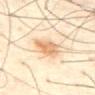follow-up — total-body-photography surveillance lesion; no biopsy | acquisition — ~15 mm crop, total-body skin-cancer survey | subject — male, aged approximately 40 | automated lesion analysis — a lesion area of about 8 mm², an outline eccentricity of about 0.55 (0 = round, 1 = elongated), and a symmetry-axis asymmetry near 0.25; a mean CIELAB color near L≈76 a*≈19 b*≈39, a lesion–skin lightness drop of about 12, and a lesion-to-skin contrast of about 7.5 (normalized; higher = more distinct); a border-irregularity index near 2.5/10, a color-variation rating of about 3.5/10, and a peripheral color-asymmetry measure near 1; a classifier nevus-likeness of about 85/100 and a detector confidence of about 100 out of 100 that the crop contains a lesion | lesion diameter — ≈3.5 mm | anatomic site — the abdomen.